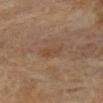Q: Was a biopsy performed?
A: imaged on a skin check; not biopsied
Q: What are the patient's age and sex?
A: female, roughly 70 years of age
Q: Lesion location?
A: the right lower leg
Q: What is the lesion's diameter?
A: about 2.5 mm
Q: How was the tile lit?
A: cross-polarized
Q: What did automated image analysis measure?
A: a mean CIELAB color near L≈43 a*≈17 b*≈29, about 5 CIELAB-L* units darker than the surrounding skin, and a normalized border contrast of about 5; a within-lesion color-variation index near 2.5/10 and peripheral color asymmetry of about 0.5
Q: What is the imaging modality?
A: total-body-photography crop, ~15 mm field of view